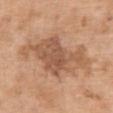Recorded during total-body skin imaging; not selected for excision or biopsy.
Located on the chest.
Longest diameter approximately 9 mm.
A 15 mm crop from a total-body photograph taken for skin-cancer surveillance.
The tile uses white-light illumination.
The subject is a female aged 53–57.